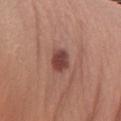notes = imaged on a skin check; not biopsied | subject = female, aged 23 to 27 | image = 15 mm crop, total-body photography | site = the left forearm.